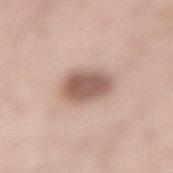Imaged with white-light lighting. The subject is a male in their 60s. A lesion tile, about 15 mm wide, cut from a 3D total-body photograph. Located on the lower back. The total-body-photography lesion software estimated a within-lesion color-variation index near 3/10 and radial color variation of about 1.